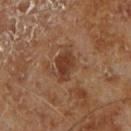Assessment:
This lesion was catalogued during total-body skin photography and was not selected for biopsy.
Context:
The patient is a male aged 68 to 72. The lesion is located on the leg. A lesion tile, about 15 mm wide, cut from a 3D total-body photograph.